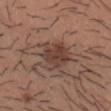Q: What is the anatomic site?
A: the chest
Q: What are the patient's age and sex?
A: male, aged approximately 30
Q: What kind of image is this?
A: ~15 mm tile from a whole-body skin photo